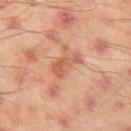Findings:
• notes — total-body-photography surveillance lesion; no biopsy
• subject — male, aged 43 to 47
• imaging modality — total-body-photography crop, ~15 mm field of view
• location — the leg
• tile lighting — cross-polarized illumination